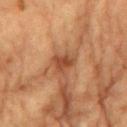follow-up = catalogued during a skin exam; not biopsied | image = ~15 mm tile from a whole-body skin photo | body site = the chest | subject = male, about 85 years old or older | diameter = ~3 mm (longest diameter).